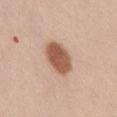Recorded during total-body skin imaging; not selected for excision or biopsy. This is a white-light tile. Measured at roughly 4 mm in maximum diameter. From the right thigh. Cropped from a whole-body photographic skin survey; the tile spans about 15 mm. The patient is a male aged approximately 60. Automated image analysis of the tile measured a color-variation rating of about 3/10 and a peripheral color-asymmetry measure near 1.